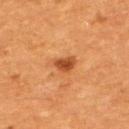{
  "biopsy_status": "not biopsied; imaged during a skin examination",
  "site": "upper back",
  "image": {
    "source": "total-body photography crop",
    "field_of_view_mm": 15
  },
  "lesion_size": {
    "long_diameter_mm_approx": 3.5
  },
  "lighting": "cross-polarized",
  "patient": {
    "sex": "male",
    "age_approx": 60
  },
  "automated_metrics": {
    "cielab_L": 48,
    "cielab_a": 27,
    "cielab_b": 40,
    "vs_skin_darker_L": 11.0,
    "vs_skin_contrast_norm": 7.5
  }
}